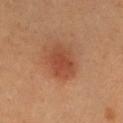Assessment: Recorded during total-body skin imaging; not selected for excision or biopsy. Background: This image is a 15 mm lesion crop taken from a total-body photograph. A female subject, aged approximately 45. The lesion is located on the abdomen. Measured at roughly 4.5 mm in maximum diameter. The total-body-photography lesion software estimated a lesion color around L≈47 a*≈26 b*≈33 in CIELAB and a lesion-to-skin contrast of about 6.5 (normalized; higher = more distinct). It also reported border irregularity of about 2 on a 0–10 scale, a within-lesion color-variation index near 3.5/10, and a peripheral color-asymmetry measure near 1. Imaged with cross-polarized lighting.